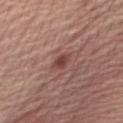Q: Was this lesion biopsied?
A: imaged on a skin check; not biopsied
Q: Automated lesion metrics?
A: an area of roughly 4 mm² and a shape eccentricity near 0.8; a border-irregularity rating of about 2/10, a within-lesion color-variation index near 2/10, and a peripheral color-asymmetry measure near 0.5; a nevus-likeness score of about 80/100 and a lesion-detection confidence of about 100/100
Q: Where on the body is the lesion?
A: the arm
Q: What kind of image is this?
A: total-body-photography crop, ~15 mm field of view
Q: What is the lesion's diameter?
A: ~3 mm (longest diameter)
Q: What lighting was used for the tile?
A: white-light
Q: What are the patient's age and sex?
A: male, about 60 years old